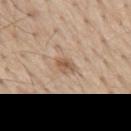Q: Is there a histopathology result?
A: no biopsy performed (imaged during a skin exam)
Q: Who is the patient?
A: male, aged approximately 70
Q: How was the tile lit?
A: white-light illumination
Q: What kind of image is this?
A: ~15 mm crop, total-body skin-cancer survey
Q: Where on the body is the lesion?
A: the mid back
Q: How large is the lesion?
A: ~2.5 mm (longest diameter)